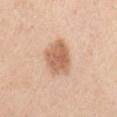Imaged during a routine full-body skin examination; the lesion was not biopsied and no histopathology is available. A female patient approximately 45 years of age. Located on the left upper arm. A 15 mm crop from a total-body photograph taken for skin-cancer surveillance.